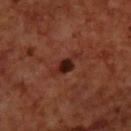Case summary:
- follow-up: catalogued during a skin exam; not biopsied
- body site: the upper back
- subject: male, approximately 70 years of age
- acquisition: ~15 mm crop, total-body skin-cancer survey
- tile lighting: cross-polarized
- automated lesion analysis: an area of roughly 4.5 mm², a shape eccentricity near 0.9, and a shape-asymmetry score of about 0.3 (0 = symmetric); border irregularity of about 4 on a 0–10 scale, a within-lesion color-variation index near 3.5/10, and a peripheral color-asymmetry measure near 1
- lesion diameter: ≈4 mm